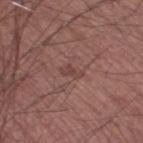This image is a 15 mm lesion crop taken from a total-body photograph.
The recorded lesion diameter is about 2.5 mm.
A male patient, aged 43 to 47.
Imaged with white-light lighting.
Located on the left thigh.